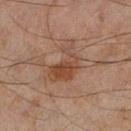- workup: imaged on a skin check; not biopsied
- size: ≈5.5 mm
- image source: ~15 mm crop, total-body skin-cancer survey
- anatomic site: the left lower leg
- subject: male, aged 43–47